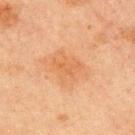Measured at roughly 3.5 mm in maximum diameter. A male subject about 65 years old. This image is a 15 mm lesion crop taken from a total-body photograph. On the front of the torso. Imaged with cross-polarized lighting. Automated image analysis of the tile measured a lesion area of about 4.5 mm², an outline eccentricity of about 0.85 (0 = round, 1 = elongated), and two-axis asymmetry of about 0.5. The analysis additionally found a lesion color around L≈50 a*≈22 b*≈35 in CIELAB and a lesion–skin lightness drop of about 5. The software also gave a border-irregularity index near 5/10, a within-lesion color-variation index near 1/10, and radial color variation of about 0.5. The software also gave a classifier nevus-likeness of about 10/100 and a detector confidence of about 100 out of 100 that the crop contains a lesion.